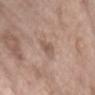Q: Was this lesion biopsied?
A: total-body-photography surveillance lesion; no biopsy